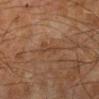Q: Was a biopsy performed?
A: imaged on a skin check; not biopsied
Q: How was the tile lit?
A: cross-polarized illumination
Q: How was this image acquired?
A: ~15 mm tile from a whole-body skin photo
Q: Lesion location?
A: the leg
Q: What is the lesion's diameter?
A: about 4 mm
Q: Who is the patient?
A: male, aged 63–67